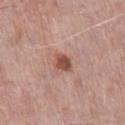notes: total-body-photography surveillance lesion; no biopsy | body site: the chest | image: 15 mm crop, total-body photography | patient: male, aged 68 to 72 | image-analysis metrics: a mean CIELAB color near L≈51 a*≈23 b*≈26 and a lesion–skin lightness drop of about 12; a border-irregularity rating of about 2/10, a within-lesion color-variation index near 4.5/10, and a peripheral color-asymmetry measure near 1.5; a lesion-detection confidence of about 100/100.